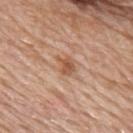The lesion was tiled from a total-body skin photograph and was not biopsied. The patient is a female in their mid-60s. The recorded lesion diameter is about 2.5 mm. This is a white-light tile. Located on the upper back. This image is a 15 mm lesion crop taken from a total-body photograph.